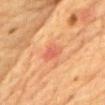Assessment: No biopsy was performed on this lesion — it was imaged during a full skin examination and was not determined to be concerning. Acquisition and patient details: From the chest. Measured at roughly 2.5 mm in maximum diameter. A male patient approximately 85 years of age or older. A 15 mm close-up extracted from a 3D total-body photography capture.